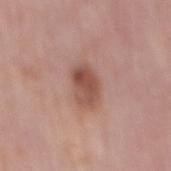Part of a total-body skin-imaging series; this lesion was reviewed on a skin check and was not flagged for biopsy. On the mid back. The lesion-visualizer software estimated an area of roughly 9 mm², an outline eccentricity of about 0.85 (0 = round, 1 = elongated), and a shape-asymmetry score of about 0.2 (0 = symmetric). And it measured a nevus-likeness score of about 85/100. A male subject, aged 73–77. Captured under white-light illumination. A lesion tile, about 15 mm wide, cut from a 3D total-body photograph.